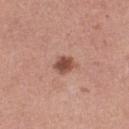anatomic site = the left thigh; image = ~15 mm crop, total-body skin-cancer survey; patient = female, approximately 30 years of age.